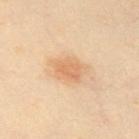biopsy_status: not biopsied; imaged during a skin examination
site: chest
image:
  source: total-body photography crop
  field_of_view_mm: 15
automated_metrics:
  vs_skin_darker_L: 9.0
  vs_skin_contrast_norm: 6.0
  border_irregularity_0_10: 3.0
  color_variation_0_10: 2.5
  peripheral_color_asymmetry: 1.0
patient:
  sex: female
  age_approx: 50
lesion_size:
  long_diameter_mm_approx: 4.5
lighting: cross-polarized